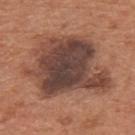notes — no biopsy performed (imaged during a skin exam)
image-analysis metrics — a lesion area of about 46 mm², a shape eccentricity near 0.6, and two-axis asymmetry of about 0.4; a border-irregularity index near 5/10, a within-lesion color-variation index near 7/10, and peripheral color asymmetry of about 1.5
acquisition — 15 mm crop, total-body photography
patient — male, in their mid- to late 60s
location — the mid back
lighting — white-light illumination
size — ≈9.5 mm